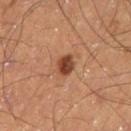biopsy status: catalogued during a skin exam; not biopsied | lesion size: ≈2.5 mm | imaging modality: ~15 mm crop, total-body skin-cancer survey | location: the left thigh | image-analysis metrics: border irregularity of about 1.5 on a 0–10 scale and radial color variation of about 1; a nevus-likeness score of about 95/100 and a detector confidence of about 100 out of 100 that the crop contains a lesion | illumination: cross-polarized | patient: male, approximately 60 years of age.